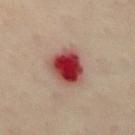notes: catalogued during a skin exam; not biopsied | location: the chest | patient: female, aged 58–62 | image source: ~15 mm crop, total-body skin-cancer survey.